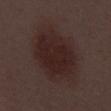| key | value |
|---|---|
| notes | no biopsy performed (imaged during a skin exam) |
| acquisition | ~15 mm crop, total-body skin-cancer survey |
| subject | male, approximately 55 years of age |
| automated metrics | an area of roughly 31 mm² and two-axis asymmetry of about 0.15; a lesion color around L≈23 a*≈16 b*≈16 in CIELAB, about 7 CIELAB-L* units darker than the surrounding skin, and a lesion-to-skin contrast of about 8.5 (normalized; higher = more distinct) |
| anatomic site | the abdomen |
| tile lighting | white-light |
| diameter | ~7.5 mm (longest diameter) |